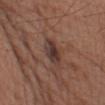notes = total-body-photography surveillance lesion; no biopsy
lesion diameter = ≈4.5 mm
image = 15 mm crop, total-body photography
automated metrics = an area of roughly 6 mm², an eccentricity of roughly 0.95, and a symmetry-axis asymmetry near 0.25; a border-irregularity index near 3/10, internal color variation of about 4 on a 0–10 scale, and a peripheral color-asymmetry measure near 1; a classifier nevus-likeness of about 0/100 and a lesion-detection confidence of about 85/100
location = the left upper arm
patient = male, aged 53–57
lighting = white-light